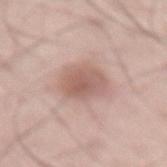| field | value |
|---|---|
| tile lighting | white-light |
| subject | male, aged 43–47 |
| image source | total-body-photography crop, ~15 mm field of view |
| body site | the lower back |